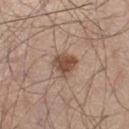Recorded during total-body skin imaging; not selected for excision or biopsy. The lesion is on the left thigh. This is a white-light tile. A 15 mm close-up tile from a total-body photography series done for melanoma screening. Measured at roughly 3.5 mm in maximum diameter. The patient is a male aged 43–47.